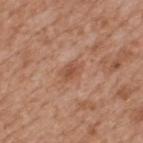Q: Is there a histopathology result?
A: total-body-photography surveillance lesion; no biopsy